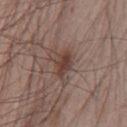notes: no biopsy performed (imaged during a skin exam)
TBP lesion metrics: an outline eccentricity of about 0.7 (0 = round, 1 = elongated); border irregularity of about 2.5 on a 0–10 scale, a within-lesion color-variation index near 4/10, and peripheral color asymmetry of about 1.5; a classifier nevus-likeness of about 90/100 and a lesion-detection confidence of about 100/100
subject: male, aged around 65
lighting: white-light illumination
body site: the chest
lesion size: ~3 mm (longest diameter)
image source: 15 mm crop, total-body photography